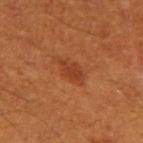Case summary:
– follow-up — no biopsy performed (imaged during a skin exam)
– anatomic site — the right upper arm
– illumination — cross-polarized
– lesion diameter — ≈3.5 mm
– patient — male, aged 58–62
– automated metrics — about 7 CIELAB-L* units darker than the surrounding skin; an automated nevus-likeness rating near 65 out of 100 and lesion-presence confidence of about 100/100
– image source — 15 mm crop, total-body photography